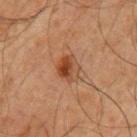This lesion was catalogued during total-body skin photography and was not selected for biopsy.
The patient is a male in their mid- to late 60s.
Automated image analysis of the tile measured a mean CIELAB color near L≈37 a*≈20 b*≈29, roughly 9 lightness units darker than nearby skin, and a normalized lesion–skin contrast near 8. The analysis additionally found a lesion-detection confidence of about 100/100.
Longest diameter approximately 3 mm.
From the left upper arm.
This is a cross-polarized tile.
A 15 mm crop from a total-body photograph taken for skin-cancer surveillance.